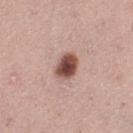workup = catalogued during a skin exam; not biopsied
location = the left thigh
image source = total-body-photography crop, ~15 mm field of view
TBP lesion metrics = a within-lesion color-variation index near 4.5/10 and a peripheral color-asymmetry measure near 1
patient = female, aged around 40
illumination = white-light
lesion diameter = ~3 mm (longest diameter)